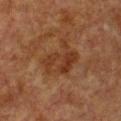workup: total-body-photography surveillance lesion; no biopsy
tile lighting: cross-polarized illumination
image source: ~15 mm tile from a whole-body skin photo
patient: female, in their mid-50s
site: the chest
size: ~5 mm (longest diameter)